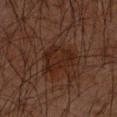<record>
<biopsy_status>not biopsied; imaged during a skin examination</biopsy_status>
<image>
  <source>total-body photography crop</source>
  <field_of_view_mm>15</field_of_view_mm>
</image>
<site>left forearm</site>
<patient>
  <sex>male</sex>
  <age_approx>65</age_approx>
</patient>
<automated_metrics>
  <cielab_L>19</cielab_L>
  <cielab_a>16</cielab_a>
  <cielab_b>20</cielab_b>
  <vs_skin_darker_L>5.0</vs_skin_darker_L>
  <vs_skin_contrast_norm>7.0</vs_skin_contrast_norm>
  <border_irregularity_0_10>3.0</border_irregularity_0_10>
  <color_variation_0_10>2.5</color_variation_0_10>
</automated_metrics>
<lesion_size>
  <long_diameter_mm_approx>4.5</long_diameter_mm_approx>
</lesion_size>
</record>